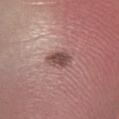workup=imaged on a skin check; not biopsied
subject=female, in their 20s
illumination=white-light
lesion diameter=~3 mm (longest diameter)
location=the left lower leg
image=total-body-photography crop, ~15 mm field of view